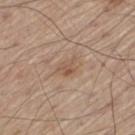follow-up: imaged on a skin check; not biopsied | anatomic site: the left thigh | subject: male, aged around 60 | acquisition: ~15 mm tile from a whole-body skin photo | tile lighting: white-light | TBP lesion metrics: a footprint of about 3.5 mm², an eccentricity of roughly 0.7, and a symmetry-axis asymmetry near 0.4; a border-irregularity rating of about 3.5/10 and peripheral color asymmetry of about 1; a classifier nevus-likeness of about 0/100 and a lesion-detection confidence of about 100/100 | size: about 2.5 mm.